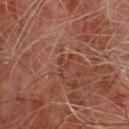Background: The lesion-visualizer software estimated an area of roughly 3 mm², a shape eccentricity near 0.85, and a shape-asymmetry score of about 0.55 (0 = symmetric). A male subject, aged 63 to 67. Longest diameter approximately 3 mm. Captured under cross-polarized illumination. A 15 mm crop from a total-body photograph taken for skin-cancer surveillance.